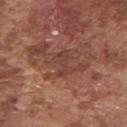lighting: white-light
patient:
  sex: male
  age_approx: 75
automated_metrics:
  area_mm2_approx: 4.5
  eccentricity: 0.9
  shape_asymmetry: 0.45
  cielab_L: 41
  cielab_a: 23
  cielab_b: 26
  vs_skin_darker_L: 6.0
  vs_skin_contrast_norm: 5.0
  peripheral_color_asymmetry: 0.0
lesion_size:
  long_diameter_mm_approx: 3.5
image:
  source: total-body photography crop
  field_of_view_mm: 15
site: right upper arm